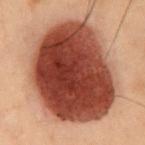Imaged during a routine full-body skin examination; the lesion was not biopsied and no histopathology is available.
Automated tile analysis of the lesion measured a mean CIELAB color near L≈36 a*≈23 b*≈26 and a lesion-to-skin contrast of about 15.5 (normalized; higher = more distinct).
The lesion is on the chest.
A male patient, aged approximately 55.
This image is a 15 mm lesion crop taken from a total-body photograph.
Measured at roughly 11.5 mm in maximum diameter.